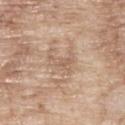Clinical impression:
The lesion was tiled from a total-body skin photograph and was not biopsied.
Clinical summary:
An algorithmic analysis of the crop reported an outline eccentricity of about 0.9 (0 = round, 1 = elongated). The software also gave a mean CIELAB color near L≈59 a*≈17 b*≈29, a lesion–skin lightness drop of about 7, and a normalized border contrast of about 4.5. The software also gave internal color variation of about 0.5 on a 0–10 scale and peripheral color asymmetry of about 0. And it measured an automated nevus-likeness rating near 0 out of 100. A male patient, aged approximately 65. The lesion's longest dimension is about 3 mm. A 15 mm close-up extracted from a 3D total-body photography capture. From the upper back. Imaged with white-light lighting.